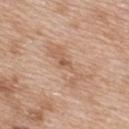The lesion was tiled from a total-body skin photograph and was not biopsied.
From the upper back.
The recorded lesion diameter is about 5.5 mm.
This is a white-light tile.
A female subject, about 40 years old.
A region of skin cropped from a whole-body photographic capture, roughly 15 mm wide.